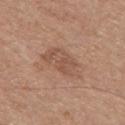The tile uses white-light illumination.
About 5 mm across.
The lesion is located on the mid back.
A male patient, aged 28–32.
A 15 mm crop from a total-body photograph taken for skin-cancer surveillance.